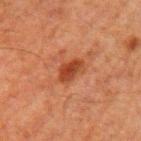Recorded during total-body skin imaging; not selected for excision or biopsy.
The subject is a male aged 58–62.
Captured under cross-polarized illumination.
About 3.5 mm across.
The lesion is on the left upper arm.
A roughly 15 mm field-of-view crop from a total-body skin photograph.